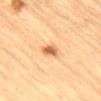Clinical impression: Part of a total-body skin-imaging series; this lesion was reviewed on a skin check and was not flagged for biopsy. Context: The lesion is located on the lower back. A male subject, aged 83 or older. Approximately 2.5 mm at its widest. Cropped from a whole-body photographic skin survey; the tile spans about 15 mm. The tile uses cross-polarized illumination.